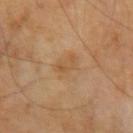Imaged during a routine full-body skin examination; the lesion was not biopsied and no histopathology is available. A region of skin cropped from a whole-body photographic capture, roughly 15 mm wide. Located on the right upper arm. A male subject, about 70 years old. The recorded lesion diameter is about 3.5 mm. Automated image analysis of the tile measured a lesion color around L≈51 a*≈17 b*≈34 in CIELAB, about 6 CIELAB-L* units darker than the surrounding skin, and a normalized lesion–skin contrast near 4.5. Captured under cross-polarized illumination.